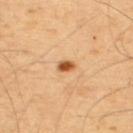  biopsy_status: not biopsied; imaged during a skin examination
  site: upper back
  patient:
    sex: male
    age_approx: 55
  automated_metrics:
    cielab_L: 55
    cielab_a: 24
    cielab_b: 41
    vs_skin_darker_L: 15.0
    vs_skin_contrast_norm: 10.5
    lesion_detection_confidence_0_100: 100
  image:
    source: total-body photography crop
    field_of_view_mm: 15
  lesion_size:
    long_diameter_mm_approx: 2.0